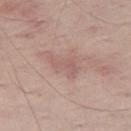follow-up: total-body-photography surveillance lesion; no biopsy | subject: male, aged 63–67 | tile lighting: white-light illumination | image: ~15 mm tile from a whole-body skin photo | body site: the right thigh | size: about 4 mm.